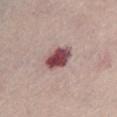This lesion was catalogued during total-body skin photography and was not selected for biopsy. A 15 mm close-up tile from a total-body photography series done for melanoma screening. A female subject, in their mid-60s. The lesion is on the chest. The tile uses white-light illumination. Longest diameter approximately 4 mm.